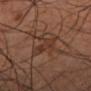{"biopsy_status": "not biopsied; imaged during a skin examination", "image": {"source": "total-body photography crop", "field_of_view_mm": 15}, "site": "left lower leg", "patient": {"sex": "male", "age_approx": 55}, "lesion_size": {"long_diameter_mm_approx": 3.0}, "lighting": "cross-polarized"}